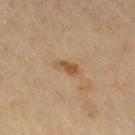A female subject aged 48–52. The recorded lesion diameter is about 3 mm. A lesion tile, about 15 mm wide, cut from a 3D total-body photograph. The lesion is located on the right thigh.